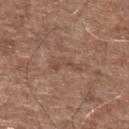Captured during whole-body skin photography for melanoma surveillance; the lesion was not biopsied.
Located on the left upper arm.
The subject is a male aged around 75.
The tile uses white-light illumination.
Cropped from a total-body skin-imaging series; the visible field is about 15 mm.